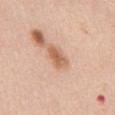Assessment: Part of a total-body skin-imaging series; this lesion was reviewed on a skin check and was not flagged for biopsy. Clinical summary: The subject is a male roughly 50 years of age. From the abdomen. Cropped from a whole-body photographic skin survey; the tile spans about 15 mm. Longest diameter approximately 3 mm. This is a white-light tile.